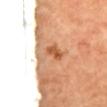| field | value |
|---|---|
| notes | catalogued during a skin exam; not biopsied |
| subject | female |
| anatomic site | the mid back |
| image source | ~15 mm crop, total-body skin-cancer survey |
| automated metrics | an area of roughly 9 mm², an eccentricity of roughly 0.7, and a shape-asymmetry score of about 0.5 (0 = symmetric); roughly 12 lightness units darker than nearby skin and a lesion-to-skin contrast of about 7.5 (normalized; higher = more distinct); a border-irregularity rating of about 5.5/10, a within-lesion color-variation index near 6.5/10, and peripheral color asymmetry of about 2 |
| lesion size | ~4.5 mm (longest diameter) |
| tile lighting | cross-polarized illumination |